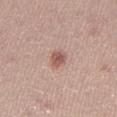Impression:
The lesion was photographed on a routine skin check and not biopsied; there is no pathology result.
Background:
The patient is a female aged around 25. Imaged with white-light lighting. On the leg. Longest diameter approximately 2.5 mm. A lesion tile, about 15 mm wide, cut from a 3D total-body photograph. The lesion-visualizer software estimated a lesion area of about 4 mm² and a shape eccentricity near 0.7. The software also gave an average lesion color of about L≈56 a*≈22 b*≈25 (CIELAB) and a lesion–skin lightness drop of about 11.